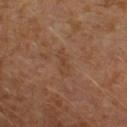The lesion was photographed on a routine skin check and not biopsied; there is no pathology result. A male patient, aged around 30. The lesion is located on the left leg. The recorded lesion diameter is about 3.5 mm. Cropped from a whole-body photographic skin survey; the tile spans about 15 mm.